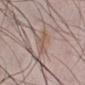Assessment: The lesion was photographed on a routine skin check and not biopsied; there is no pathology result.